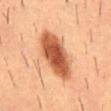Notes:
– follow-up · catalogued during a skin exam; not biopsied
– body site · the lower back
– image source · 15 mm crop, total-body photography
– subject · male, about 55 years old
– lighting · cross-polarized
– lesion diameter · ~7 mm (longest diameter)